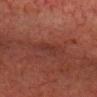  image:
    source: total-body photography crop
    field_of_view_mm: 15
  lighting: cross-polarized
  automated_metrics:
    area_mm2_approx: 3.0
    eccentricity: 0.85
    border_irregularity_0_10: 4.5
    color_variation_0_10: 0.0
    peripheral_color_asymmetry: 0.0
  patient:
    sex: male
    age_approx: 75
  site: chest
  lesion_size:
    long_diameter_mm_approx: 2.5